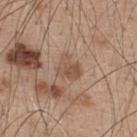Q: Is there a histopathology result?
A: catalogued during a skin exam; not biopsied
Q: What is the imaging modality?
A: ~15 mm crop, total-body skin-cancer survey
Q: Who is the patient?
A: male, roughly 50 years of age
Q: Lesion location?
A: the upper back